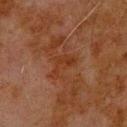The subject is a male about 80 years old. The lesion-visualizer software estimated a lesion area of about 4.5 mm², an outline eccentricity of about 0.8 (0 = round, 1 = elongated), and a shape-asymmetry score of about 0.7 (0 = symmetric). Located on the upper back. A roughly 15 mm field-of-view crop from a total-body skin photograph.